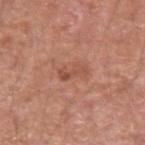• workup — no biopsy performed (imaged during a skin exam)
• body site — the left upper arm
• subject — male, aged 53 to 57
• acquisition — 15 mm crop, total-body photography